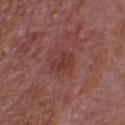{
  "lesion_size": {
    "long_diameter_mm_approx": 3.0
  },
  "site": "chest",
  "patient": {
    "sex": "male",
    "age_approx": 65
  },
  "automated_metrics": {
    "area_mm2_approx": 4.5,
    "eccentricity": 0.85,
    "shape_asymmetry": 0.25,
    "border_irregularity_0_10": 2.5,
    "color_variation_0_10": 1.5
  },
  "lighting": "white-light",
  "image": {
    "source": "total-body photography crop",
    "field_of_view_mm": 15
  }
}